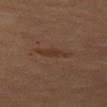Clinical impression:
The lesion was photographed on a routine skin check and not biopsied; there is no pathology result.
Context:
Approximately 4.5 mm at its widest. The lesion is on the left thigh. Captured under cross-polarized illumination. This image is a 15 mm lesion crop taken from a total-body photograph. A female subject aged around 50.